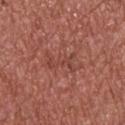subject=male, about 65 years old | lesion size=≈3.5 mm | site=the upper back | imaging modality=15 mm crop, total-body photography.